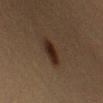Assessment: Imaged during a routine full-body skin examination; the lesion was not biopsied and no histopathology is available. Background: Cropped from a total-body skin-imaging series; the visible field is about 15 mm. A male subject, aged 38 to 42. The lesion is located on the arm. Longest diameter approximately 3.5 mm. An algorithmic analysis of the crop reported a lesion area of about 6 mm², an eccentricity of roughly 0.8, and a shape-asymmetry score of about 0.15 (0 = symmetric). The software also gave a classifier nevus-likeness of about 95/100 and lesion-presence confidence of about 100/100.